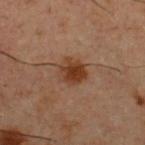biopsy status = imaged on a skin check; not biopsied
image = 15 mm crop, total-body photography
subject = male, in their 60s
illumination = cross-polarized
body site = the chest
lesion diameter = about 3 mm